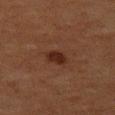Captured during whole-body skin photography for melanoma surveillance; the lesion was not biopsied. From the left thigh. A 15 mm close-up extracted from a 3D total-body photography capture. About 2.5 mm across. The patient is a female about 50 years old. The lesion-visualizer software estimated a lesion area of about 4 mm² and a symmetry-axis asymmetry near 0.2. The software also gave a mean CIELAB color near L≈21 a*≈18 b*≈22, about 8 CIELAB-L* units darker than the surrounding skin, and a normalized border contrast of about 9. The analysis additionally found a border-irregularity index near 2/10 and a within-lesion color-variation index near 2/10. The analysis additionally found an automated nevus-likeness rating near 80 out of 100.